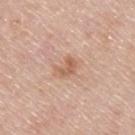No biopsy was performed on this lesion — it was imaged during a full skin examination and was not determined to be concerning. A 15 mm crop from a total-body photograph taken for skin-cancer surveillance. A male patient, aged 43–47. On the upper back. Longest diameter approximately 2.5 mm.